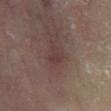Part of a total-body skin-imaging series; this lesion was reviewed on a skin check and was not flagged for biopsy.
The lesion is on the left lower leg.
This image is a 15 mm lesion crop taken from a total-body photograph.
The subject is a male aged 63 to 67.